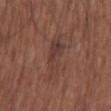Notes:
- imaging modality · ~15 mm crop, total-body skin-cancer survey
- subject · female, aged approximately 75
- illumination · white-light
- automated metrics · an area of roughly 9.5 mm², a shape eccentricity near 0.95, and a symmetry-axis asymmetry near 0.25; an average lesion color of about L≈38 a*≈20 b*≈22 (CIELAB), about 6 CIELAB-L* units darker than the surrounding skin, and a lesion-to-skin contrast of about 6.5 (normalized; higher = more distinct); a border-irregularity index near 4.5/10, a within-lesion color-variation index near 4.5/10, and peripheral color asymmetry of about 1.5
- site · the head or neck